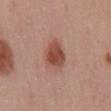Impression: Recorded during total-body skin imaging; not selected for excision or biopsy. Image and clinical context: The total-body-photography lesion software estimated a mean CIELAB color near L≈48 a*≈26 b*≈28, about 12 CIELAB-L* units darker than the surrounding skin, and a lesion-to-skin contrast of about 9 (normalized; higher = more distinct). And it measured a nevus-likeness score of about 100/100 and lesion-presence confidence of about 100/100. Approximately 4 mm at its widest. A male subject, approximately 60 years of age. Captured under white-light illumination. From the chest. A roughly 15 mm field-of-view crop from a total-body skin photograph.